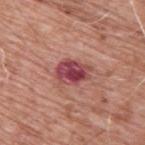No biopsy was performed on this lesion — it was imaged during a full skin examination and was not determined to be concerning.
A region of skin cropped from a whole-body photographic capture, roughly 15 mm wide.
The recorded lesion diameter is about 4 mm.
A male patient, aged 58–62.
The lesion is on the upper back.
This is a white-light tile.